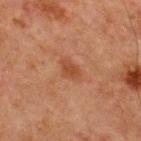biopsy status: catalogued during a skin exam; not biopsied
imaging modality: ~15 mm crop, total-body skin-cancer survey
body site: the chest
subject: male, aged approximately 65
illumination: cross-polarized illumination